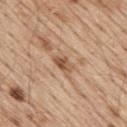image source — ~15 mm crop, total-body skin-cancer survey | TBP lesion metrics — a lesion area of about 4 mm² and an outline eccentricity of about 0.8 (0 = round, 1 = elongated); a lesion–skin lightness drop of about 11 and a normalized lesion–skin contrast near 7.5; a nevus-likeness score of about 0/100 | lesion diameter — about 3 mm | tile lighting — white-light | subject — male, aged 68–72 | location — the back.